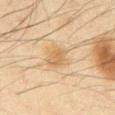biopsy status = total-body-photography surveillance lesion; no biopsy | TBP lesion metrics = a lesion area of about 5 mm², an eccentricity of roughly 0.7, and two-axis asymmetry of about 0.2; an average lesion color of about L≈52 a*≈14 b*≈33 (CIELAB), roughly 7 lightness units darker than nearby skin, and a normalized border contrast of about 6; an automated nevus-likeness rating near 65 out of 100 | illumination = cross-polarized | size = about 3 mm | patient = male, roughly 65 years of age | body site = the abdomen | acquisition = total-body-photography crop, ~15 mm field of view.